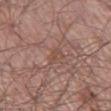Recorded during total-body skin imaging; not selected for excision or biopsy. Located on the left thigh. The total-body-photography lesion software estimated a classifier nevus-likeness of about 0/100 and lesion-presence confidence of about 85/100. The patient is a male in their mid-50s. This is a white-light tile. Measured at roughly 2.5 mm in maximum diameter. A region of skin cropped from a whole-body photographic capture, roughly 15 mm wide.